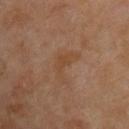Acquisition and patient details:
A male patient, roughly 65 years of age. A lesion tile, about 15 mm wide, cut from a 3D total-body photograph. On the upper back. The tile uses cross-polarized illumination. The recorded lesion diameter is about 4.5 mm. The total-body-photography lesion software estimated an outline eccentricity of about 0.85 (0 = round, 1 = elongated) and a symmetry-axis asymmetry near 0.65. And it measured roughly 5 lightness units darker than nearby skin and a normalized border contrast of about 5.5. It also reported a border-irregularity index near 7.5/10, internal color variation of about 1.5 on a 0–10 scale, and radial color variation of about 0.5. The software also gave a nevus-likeness score of about 0/100.